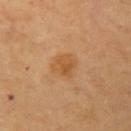Recorded during total-body skin imaging; not selected for excision or biopsy.
The lesion's longest dimension is about 3 mm.
The subject is aged 58 to 62.
This is a cross-polarized tile.
From the left upper arm.
Automated image analysis of the tile measured a lesion color around L≈54 a*≈21 b*≈40 in CIELAB, about 7 CIELAB-L* units darker than the surrounding skin, and a normalized border contrast of about 6.5. The analysis additionally found a classifier nevus-likeness of about 25/100 and a detector confidence of about 100 out of 100 that the crop contains a lesion.
A roughly 15 mm field-of-view crop from a total-body skin photograph.